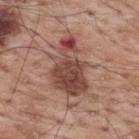| field | value |
|---|---|
| workup | imaged on a skin check; not biopsied |
| patient | male, aged 68 to 72 |
| image | total-body-photography crop, ~15 mm field of view |
| body site | the upper back |